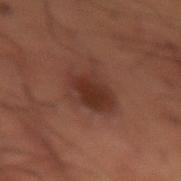Captured during whole-body skin photography for melanoma surveillance; the lesion was not biopsied.
The lesion is on the right thigh.
Cropped from a total-body skin-imaging series; the visible field is about 15 mm.
Imaged with cross-polarized lighting.
The patient is a male aged around 50.